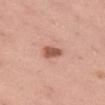Q: Was a biopsy performed?
A: total-body-photography surveillance lesion; no biopsy
Q: What lighting was used for the tile?
A: white-light
Q: Lesion location?
A: the left thigh
Q: Lesion size?
A: ~2.5 mm (longest diameter)
Q: Patient demographics?
A: female, about 45 years old
Q: Automated lesion metrics?
A: a lesion color around L≈56 a*≈25 b*≈30 in CIELAB, roughly 13 lightness units darker than nearby skin, and a lesion-to-skin contrast of about 9 (normalized; higher = more distinct); a border-irregularity index near 1.5/10, a color-variation rating of about 2/10, and a peripheral color-asymmetry measure near 0.5; a detector confidence of about 100 out of 100 that the crop contains a lesion
Q: What is the imaging modality?
A: 15 mm crop, total-body photography